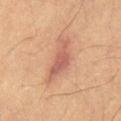  image:
    source: total-body photography crop
    field_of_view_mm: 15
  automated_metrics:
    area_mm2_approx: 10.0
    shape_asymmetry: 0.45
    cielab_L: 51
    cielab_a: 22
    cielab_b: 27
    vs_skin_darker_L: 9.0
    vs_skin_contrast_norm: 7.0
    border_irregularity_0_10: 5.5
    peripheral_color_asymmetry: 1.0
  patient:
    sex: male
    age_approx: 60
  lesion_size:
    long_diameter_mm_approx: 6.0
  lighting: cross-polarized